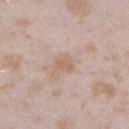biopsy status = no biopsy performed (imaged during a skin exam)
lighting = white-light illumination
automated metrics = a lesion color around L≈62 a*≈16 b*≈27 in CIELAB, roughly 7 lightness units darker than nearby skin, and a normalized border contrast of about 5.5
image source = ~15 mm tile from a whole-body skin photo
patient = female, aged 23–27
size = ≈2.5 mm
anatomic site = the left lower leg